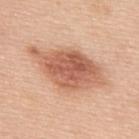Findings:
– follow-up — no biopsy performed (imaged during a skin exam)
– body site — the upper back
– acquisition — total-body-photography crop, ~15 mm field of view
– diameter — ~8.5 mm (longest diameter)
– TBP lesion metrics — a lesion area of about 27 mm² and an eccentricity of roughly 0.85; a border-irregularity index near 2.5/10, a within-lesion color-variation index near 5.5/10, and a peripheral color-asymmetry measure near 1.5
– patient — male, approximately 50 years of age